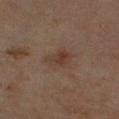Impression: Recorded during total-body skin imaging; not selected for excision or biopsy.